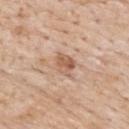Impression: Part of a total-body skin-imaging series; this lesion was reviewed on a skin check and was not flagged for biopsy. Clinical summary: Automated image analysis of the tile measured an area of roughly 5.5 mm², an outline eccentricity of about 0.65 (0 = round, 1 = elongated), and two-axis asymmetry of about 0.2. The software also gave a lesion color around L≈59 a*≈20 b*≈31 in CIELAB, about 10 CIELAB-L* units darker than the surrounding skin, and a normalized border contrast of about 7. It also reported a border-irregularity index near 2/10, internal color variation of about 4.5 on a 0–10 scale, and a peripheral color-asymmetry measure near 1.5. The software also gave a lesion-detection confidence of about 100/100. From the upper back. The subject is a male aged around 85 or older. The recorded lesion diameter is about 3 mm. Captured under white-light illumination. A roughly 15 mm field-of-view crop from a total-body skin photograph.